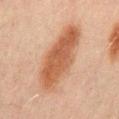Assessment:
This lesion was catalogued during total-body skin photography and was not selected for biopsy.
Acquisition and patient details:
Located on the mid back. Imaged with cross-polarized lighting. Automated tile analysis of the lesion measured a lesion color around L≈47 a*≈20 b*≈30 in CIELAB, roughly 11 lightness units darker than nearby skin, and a normalized border contrast of about 8.5. It also reported a border-irregularity index near 2/10 and a within-lesion color-variation index near 4/10. A 15 mm crop from a total-body photograph taken for skin-cancer surveillance. The recorded lesion diameter is about 9 mm. The patient is a male roughly 45 years of age.